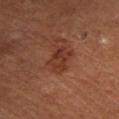| field | value |
|---|---|
| biopsy status | imaged on a skin check; not biopsied |
| body site | the leg |
| patient | male, about 55 years old |
| imaging modality | ~15 mm tile from a whole-body skin photo |
| automated lesion analysis | border irregularity of about 3 on a 0–10 scale, a within-lesion color-variation index near 4/10, and radial color variation of about 1.5; a nevus-likeness score of about 20/100 |